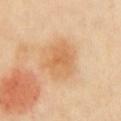follow-up: total-body-photography surveillance lesion; no biopsy | patient: female, roughly 40 years of age | lesion diameter: ~4.5 mm (longest diameter) | lighting: cross-polarized illumination | image source: ~15 mm crop, total-body skin-cancer survey | site: the chest | automated metrics: an average lesion color of about L≈67 a*≈22 b*≈41 (CIELAB), about 8 CIELAB-L* units darker than the surrounding skin, and a normalized lesion–skin contrast near 6; a border-irregularity index near 3/10 and radial color variation of about 1; a classifier nevus-likeness of about 65/100 and a detector confidence of about 100 out of 100 that the crop contains a lesion.